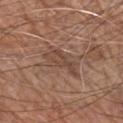Impression: Captured during whole-body skin photography for melanoma surveillance; the lesion was not biopsied. Context: A 15 mm crop from a total-body photograph taken for skin-cancer surveillance. Measured at roughly 5 mm in maximum diameter. The lesion is on the arm. A male patient roughly 70 years of age.